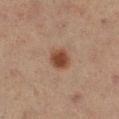Case summary:
– workup: catalogued during a skin exam; not biopsied
– TBP lesion metrics: an area of roughly 5 mm², an outline eccentricity of about 0.55 (0 = round, 1 = elongated), and two-axis asymmetry of about 0.2; an average lesion color of about L≈34 a*≈18 b*≈25 (CIELAB), roughly 11 lightness units darker than nearby skin, and a normalized lesion–skin contrast near 10.5; a nevus-likeness score of about 100/100
– acquisition: ~15 mm tile from a whole-body skin photo
– lesion size: ≈2.5 mm
– subject: female, in their mid- to late 50s
– anatomic site: the right lower leg
– tile lighting: cross-polarized illumination